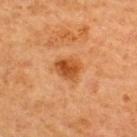biopsy status = catalogued during a skin exam; not biopsied
image = 15 mm crop, total-body photography
anatomic site = the upper back
subject = male, about 65 years old
lesion diameter = about 3.5 mm